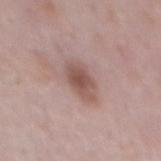The lesion was photographed on a routine skin check and not biopsied; there is no pathology result.
Imaged with white-light lighting.
The total-body-photography lesion software estimated a mean CIELAB color near L≈53 a*≈20 b*≈22 and a lesion-to-skin contrast of about 7.5 (normalized; higher = more distinct). And it measured border irregularity of about 2.5 on a 0–10 scale, a color-variation rating of about 3.5/10, and peripheral color asymmetry of about 1. The software also gave a classifier nevus-likeness of about 80/100.
The patient is a male in their 50s.
A 15 mm close-up tile from a total-body photography series done for melanoma screening.
From the back.
About 4.5 mm across.